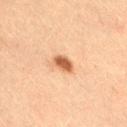Case summary:
* workup: no biopsy performed (imaged during a skin exam)
* location: the leg
* lesion size: ~2.5 mm (longest diameter)
* acquisition: total-body-photography crop, ~15 mm field of view
* patient: female, about 20 years old
* lighting: cross-polarized